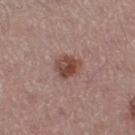This lesion was catalogued during total-body skin photography and was not selected for biopsy. Cropped from a whole-body photographic skin survey; the tile spans about 15 mm. Longest diameter approximately 3 mm. Automated image analysis of the tile measured a shape eccentricity near 0.45 and a shape-asymmetry score of about 0.25 (0 = symmetric). The lesion is located on the left thigh. A female subject in their mid- to late 50s. Captured under white-light illumination.